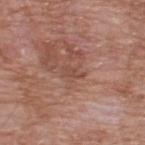illumination: white-light illumination; acquisition: total-body-photography crop, ~15 mm field of view; patient: male, aged 58–62; body site: the upper back; lesion size: ~2.5 mm (longest diameter); TBP lesion metrics: a footprint of about 2.5 mm², an outline eccentricity of about 0.9 (0 = round, 1 = elongated), and a symmetry-axis asymmetry near 0.3.